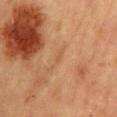No biopsy was performed on this lesion — it was imaged during a full skin examination and was not determined to be concerning. The patient is a female approximately 60 years of age. Captured under cross-polarized illumination. The recorded lesion diameter is about 1 mm. From the mid back. Cropped from a whole-body photographic skin survey; the tile spans about 15 mm.